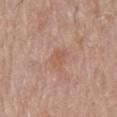Imaged during a routine full-body skin examination; the lesion was not biopsied and no histopathology is available. The lesion-visualizer software estimated a lesion area of about 5 mm² and an outline eccentricity of about 0.8 (0 = round, 1 = elongated). The analysis additionally found a classifier nevus-likeness of about 0/100 and lesion-presence confidence of about 100/100. This is a white-light tile. A 15 mm crop from a total-body photograph taken for skin-cancer surveillance. The patient is a male aged around 80. The lesion is located on the mid back.